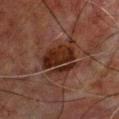follow-up — total-body-photography surveillance lesion; no biopsy | subject — male, approximately 65 years of age | site — the chest | illumination — cross-polarized illumination | imaging modality — 15 mm crop, total-body photography | size — ≈5 mm.